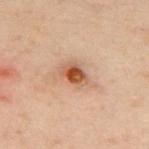Captured during whole-body skin photography for melanoma surveillance; the lesion was not biopsied.
A 15 mm close-up tile from a total-body photography series done for melanoma screening.
From the back.
A male subject, in their 50s.
The lesion-visualizer software estimated an eccentricity of roughly 0.75 and a symmetry-axis asymmetry near 0.25. It also reported an automated nevus-likeness rating near 90 out of 100 and a detector confidence of about 100 out of 100 that the crop contains a lesion.